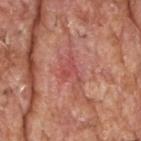<record>
<biopsy_status>not biopsied; imaged during a skin examination</biopsy_status>
<site>head or neck</site>
<patient>
  <sex>male</sex>
  <age_approx>60</age_approx>
</patient>
<image>
  <source>total-body photography crop</source>
  <field_of_view_mm>15</field_of_view_mm>
</image>
<lesion_size>
  <long_diameter_mm_approx>4.0</long_diameter_mm_approx>
</lesion_size>
<lighting>white-light</lighting>
</record>